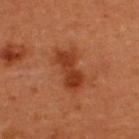Recorded during total-body skin imaging; not selected for excision or biopsy.
A region of skin cropped from a whole-body photographic capture, roughly 15 mm wide.
A female subject about 50 years old.
The lesion's longest dimension is about 5 mm.